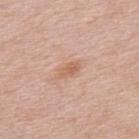{"biopsy_status": "not biopsied; imaged during a skin examination", "image": {"source": "total-body photography crop", "field_of_view_mm": 15}, "lighting": "white-light", "lesion_size": {"long_diameter_mm_approx": 2.5}, "automated_metrics": {"cielab_L": 61, "cielab_a": 22, "cielab_b": 32, "vs_skin_darker_L": 8.0, "vs_skin_contrast_norm": 6.0, "color_variation_0_10": 2.0, "peripheral_color_asymmetry": 0.5}, "patient": {"sex": "male", "age_approx": 55}, "site": "upper back"}